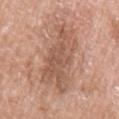Impression:
Imaged during a routine full-body skin examination; the lesion was not biopsied and no histopathology is available.
Acquisition and patient details:
A female patient aged around 60. The recorded lesion diameter is about 8 mm. This is a white-light tile. A roughly 15 mm field-of-view crop from a total-body skin photograph. From the left upper arm.